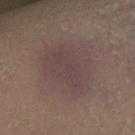<lesion>
  <biopsy_status>not biopsied; imaged during a skin examination</biopsy_status>
  <image>
    <source>total-body photography crop</source>
    <field_of_view_mm>15</field_of_view_mm>
  </image>
  <site>right lower leg</site>
  <patient>
    <sex>female</sex>
    <age_approx>20</age_approx>
  </patient>
</lesion>